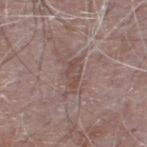No biopsy was performed on this lesion — it was imaged during a full skin examination and was not determined to be concerning. A male patient, aged around 65. This is a white-light tile. The lesion is on the back. Cropped from a whole-body photographic skin survey; the tile spans about 15 mm. Measured at roughly 3 mm in maximum diameter.